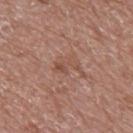Findings:
– workup: total-body-photography surveillance lesion; no biopsy
– image source: ~15 mm crop, total-body skin-cancer survey
– image-analysis metrics: lesion-presence confidence of about 100/100
– patient: male, aged 68–72
– lighting: white-light
– lesion size: ~3 mm (longest diameter)
– site: the upper back